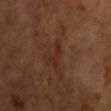Assessment:
The lesion was photographed on a routine skin check and not biopsied; there is no pathology result.
Background:
Imaged with cross-polarized lighting. The subject is a male in their mid-60s. Automated tile analysis of the lesion measured a border-irregularity index near 5/10, internal color variation of about 0.5 on a 0–10 scale, and a peripheral color-asymmetry measure near 0. And it measured a lesion-detection confidence of about 100/100. Located on the chest. A region of skin cropped from a whole-body photographic capture, roughly 15 mm wide. Longest diameter approximately 3 mm.